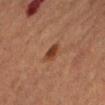Part of a total-body skin-imaging series; this lesion was reviewed on a skin check and was not flagged for biopsy. On the right forearm. Cropped from a whole-body photographic skin survey; the tile spans about 15 mm. A female patient, about 70 years old. The lesion-visualizer software estimated an area of roughly 4 mm² and a shape eccentricity near 0.8. The software also gave a border-irregularity index near 2.5/10 and radial color variation of about 0.5. The software also gave a nevus-likeness score of about 95/100 and a detector confidence of about 100 out of 100 that the crop contains a lesion.